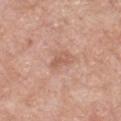Notes:
• biopsy status — no biopsy performed (imaged during a skin exam)
• site — the chest
• image source — ~15 mm crop, total-body skin-cancer survey
• illumination — white-light illumination
• lesion diameter — ~3 mm (longest diameter)
• image-analysis metrics — a footprint of about 4 mm²; a lesion color around L≈59 a*≈22 b*≈29 in CIELAB, a lesion–skin lightness drop of about 8, and a normalized lesion–skin contrast near 5.5
• patient — male, aged approximately 60